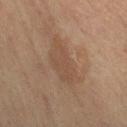This lesion was catalogued during total-body skin photography and was not selected for biopsy. The lesion is on the leg. A 15 mm close-up extracted from a 3D total-body photography capture. The patient is a female aged around 65. An algorithmic analysis of the crop reported a footprint of about 12 mm², a shape eccentricity near 0.9, and a symmetry-axis asymmetry near 0.25. The software also gave a nevus-likeness score of about 0/100 and a detector confidence of about 100 out of 100 that the crop contains a lesion. This is a cross-polarized tile. Longest diameter approximately 6 mm.